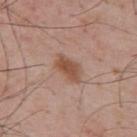<lesion>
  <biopsy_status>not biopsied; imaged during a skin examination</biopsy_status>
  <image>
    <source>total-body photography crop</source>
    <field_of_view_mm>15</field_of_view_mm>
  </image>
  <automated_metrics>
    <eccentricity>0.85</eccentricity>
    <shape_asymmetry>0.25</shape_asymmetry>
    <cielab_L>51</cielab_L>
    <cielab_a>20</cielab_a>
    <cielab_b>29</cielab_b>
    <vs_skin_contrast_norm>8.0</vs_skin_contrast_norm>
    <border_irregularity_0_10>3.0</border_irregularity_0_10>
    <peripheral_color_asymmetry>1.0</peripheral_color_asymmetry>
    <nevus_likeness_0_100>90</nevus_likeness_0_100>
    <lesion_detection_confidence_0_100>100</lesion_detection_confidence_0_100>
  </automated_metrics>
  <lesion_size>
    <long_diameter_mm_approx>4.0</long_diameter_mm_approx>
  </lesion_size>
  <patient>
    <sex>male</sex>
    <age_approx>55</age_approx>
  </patient>
  <site>upper back</site>
</lesion>